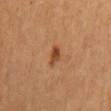Q: Was this lesion biopsied?
A: total-body-photography surveillance lesion; no biopsy
Q: Who is the patient?
A: female
Q: Where on the body is the lesion?
A: the mid back
Q: What is the imaging modality?
A: 15 mm crop, total-body photography